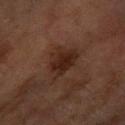workup = no biopsy performed (imaged during a skin exam) | tile lighting = cross-polarized | patient = female, roughly 60 years of age | site = the right forearm | lesion diameter = ~4.5 mm (longest diameter) | image-analysis metrics = an area of roughly 11 mm² and a shape eccentricity near 0.65; border irregularity of about 4 on a 0–10 scale, a within-lesion color-variation index near 3/10, and a peripheral color-asymmetry measure near 1 | acquisition = ~15 mm tile from a whole-body skin photo.